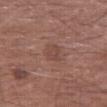| field | value |
|---|---|
| follow-up | catalogued during a skin exam; not biopsied |
| patient | male, roughly 65 years of age |
| diameter | ~3 mm (longest diameter) |
| acquisition | 15 mm crop, total-body photography |
| anatomic site | the leg |
| tile lighting | white-light |